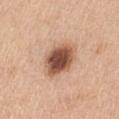Assessment:
Imaged during a routine full-body skin examination; the lesion was not biopsied and no histopathology is available.
Context:
About 5 mm across. Automated tile analysis of the lesion measured a shape eccentricity near 0.7 and two-axis asymmetry of about 0.15. The software also gave an average lesion color of about L≈53 a*≈22 b*≈30 (CIELAB), roughly 19 lightness units darker than nearby skin, and a normalized border contrast of about 12. It also reported a border-irregularity rating of about 1.5/10, a color-variation rating of about 6/10, and radial color variation of about 1.5. The software also gave a classifier nevus-likeness of about 100/100 and lesion-presence confidence of about 100/100. This is a white-light tile. The patient is a female in their mid-50s. Located on the abdomen. Cropped from a whole-body photographic skin survey; the tile spans about 15 mm.